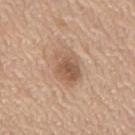Clinical impression:
The lesion was photographed on a routine skin check and not biopsied; there is no pathology result.
Image and clinical context:
From the mid back. Cropped from a total-body skin-imaging series; the visible field is about 15 mm. Automated tile analysis of the lesion measured a nevus-likeness score of about 35/100 and lesion-presence confidence of about 100/100. A male subject about 65 years old. Captured under white-light illumination. The recorded lesion diameter is about 4.5 mm.